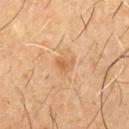{"biopsy_status": "not biopsied; imaged during a skin examination", "site": "chest", "lighting": "cross-polarized", "patient": {"sex": "male", "age_approx": 60}, "image": {"source": "total-body photography crop", "field_of_view_mm": 15}}